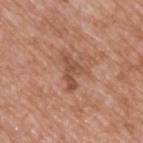Findings:
• follow-up — no biopsy performed (imaged during a skin exam)
• patient — male, aged approximately 50
• illumination — white-light
• diameter — ≈4.5 mm
• acquisition — ~15 mm crop, total-body skin-cancer survey
• automated lesion analysis — an automated nevus-likeness rating near 0 out of 100 and lesion-presence confidence of about 100/100
• anatomic site — the upper back